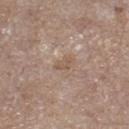Captured during whole-body skin photography for melanoma surveillance; the lesion was not biopsied.
About 2.5 mm across.
Located on the right lower leg.
This is a white-light tile.
Cropped from a whole-body photographic skin survey; the tile spans about 15 mm.
A female subject, in their mid- to late 60s.